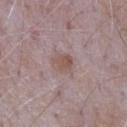  biopsy_status: not biopsied; imaged during a skin examination
  patient:
    sex: male
    age_approx: 65
  site: chest
  image:
    source: total-body photography crop
    field_of_view_mm: 15